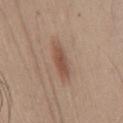Assessment:
Recorded during total-body skin imaging; not selected for excision or biopsy.
Clinical summary:
The lesion is on the chest. A 15 mm close-up extracted from a 3D total-body photography capture. A male patient, aged 43–47. Imaged with white-light lighting.